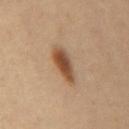subject = male, roughly 60 years of age; size = ~4.5 mm (longest diameter); image source = total-body-photography crop, ~15 mm field of view; body site = the mid back.